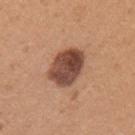Imaged during a routine full-body skin examination; the lesion was not biopsied and no histopathology is available. Captured under white-light illumination. Located on the left upper arm. A roughly 15 mm field-of-view crop from a total-body skin photograph. The lesion's longest dimension is about 4.5 mm. A female patient aged 43 to 47. The total-body-photography lesion software estimated an area of roughly 15 mm², an outline eccentricity of about 0.6 (0 = round, 1 = elongated), and a shape-asymmetry score of about 0.15 (0 = symmetric). The software also gave roughly 17 lightness units darker than nearby skin and a normalized border contrast of about 12.